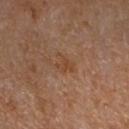workup: no biopsy performed (imaged during a skin exam)
acquisition: ~15 mm tile from a whole-body skin photo
subject: male, in their mid-60s
illumination: cross-polarized
anatomic site: the right upper arm
automated metrics: a mean CIELAB color near L≈43 a*≈20 b*≈32; border irregularity of about 5.5 on a 0–10 scale, internal color variation of about 1 on a 0–10 scale, and radial color variation of about 0.5; an automated nevus-likeness rating near 10 out of 100